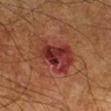<case>
<biopsy_status>not biopsied; imaged during a skin examination</biopsy_status>
<lighting>cross-polarized</lighting>
<image>
  <source>total-body photography crop</source>
  <field_of_view_mm>15</field_of_view_mm>
</image>
<lesion_size>
  <long_diameter_mm_approx>5.5</long_diameter_mm_approx>
</lesion_size>
<patient>
  <sex>male</sex>
  <age_approx>60</age_approx>
</patient>
<site>right lower leg</site>
</case>